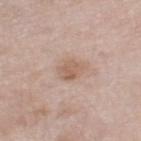Q: Was this lesion biopsied?
A: total-body-photography surveillance lesion; no biopsy
Q: What kind of image is this?
A: ~15 mm tile from a whole-body skin photo
Q: Patient demographics?
A: male, approximately 80 years of age
Q: How was the tile lit?
A: white-light illumination
Q: Where on the body is the lesion?
A: the right lower leg
Q: What is the lesion's diameter?
A: ≈2.5 mm